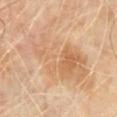The lesion was photographed on a routine skin check and not biopsied; there is no pathology result. Imaged with cross-polarized lighting. Measured at roughly 10 mm in maximum diameter. A male patient aged around 70. Cropped from a total-body skin-imaging series; the visible field is about 15 mm. The lesion is on the chest.